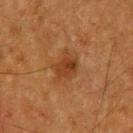Part of a total-body skin-imaging series; this lesion was reviewed on a skin check and was not flagged for biopsy. Measured at roughly 3.5 mm in maximum diameter. A 15 mm crop from a total-body photograph taken for skin-cancer surveillance. The lesion is on the back. Automated image analysis of the tile measured a lesion area of about 7 mm², an eccentricity of roughly 0.55, and two-axis asymmetry of about 0.4. The software also gave a classifier nevus-likeness of about 50/100 and a detector confidence of about 100 out of 100 that the crop contains a lesion. A male patient, aged 63 to 67. This is a cross-polarized tile.